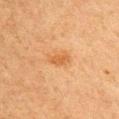Q: Was this lesion biopsied?
A: no biopsy performed (imaged during a skin exam)
Q: Lesion location?
A: the front of the torso
Q: How was this image acquired?
A: 15 mm crop, total-body photography
Q: Automated lesion metrics?
A: an area of roughly 4 mm²; a nevus-likeness score of about 25/100 and lesion-presence confidence of about 100/100
Q: What is the lesion's diameter?
A: about 3 mm
Q: Who is the patient?
A: male, aged approximately 75